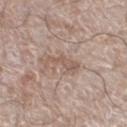Findings:
* notes — total-body-photography surveillance lesion; no biopsy
* body site — the left lower leg
* image source — ~15 mm tile from a whole-body skin photo
* subject — male, aged approximately 60
* lighting — white-light